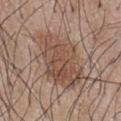biopsy status: total-body-photography surveillance lesion; no biopsy | subject: male, in their mid- to late 50s | imaging modality: 15 mm crop, total-body photography | body site: the chest | lesion diameter: ~7 mm (longest diameter) | lighting: white-light.